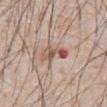notes: catalogued during a skin exam; not biopsied | size: ≈3.5 mm | acquisition: 15 mm crop, total-body photography | anatomic site: the chest | automated lesion analysis: an eccentricity of roughly 0.8 and a symmetry-axis asymmetry near 0.4; an average lesion color of about L≈55 a*≈20 b*≈25 (CIELAB), a lesion–skin lightness drop of about 11, and a lesion-to-skin contrast of about 7.5 (normalized; higher = more distinct); a peripheral color-asymmetry measure near 2 | patient: male, roughly 65 years of age | tile lighting: white-light illumination.